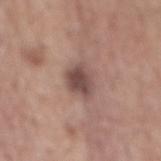The lesion was photographed on a routine skin check and not biopsied; there is no pathology result. A close-up tile cropped from a whole-body skin photograph, about 15 mm across. The total-body-photography lesion software estimated roughly 12 lightness units darker than nearby skin and a lesion-to-skin contrast of about 9 (normalized; higher = more distinct). It also reported border irregularity of about 2.5 on a 0–10 scale and radial color variation of about 1.5. From the mid back. The subject is a male aged approximately 55.